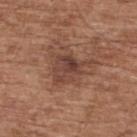No biopsy was performed on this lesion — it was imaged during a full skin examination and was not determined to be concerning. Automated image analysis of the tile measured a footprint of about 11 mm², a shape eccentricity near 0.6, and two-axis asymmetry of about 0.35. It also reported a mean CIELAB color near L≈43 a*≈21 b*≈26, about 9 CIELAB-L* units darker than the surrounding skin, and a normalized border contrast of about 7. And it measured a nevus-likeness score of about 0/100. This is a white-light tile. This image is a 15 mm lesion crop taken from a total-body photograph. From the back. Approximately 4.5 mm at its widest. A male patient, about 75 years old.